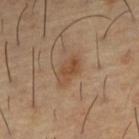Captured during whole-body skin photography for melanoma surveillance; the lesion was not biopsied.
A male subject, about 55 years old.
The lesion is on the right forearm.
A 15 mm close-up tile from a total-body photography series done for melanoma screening.